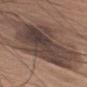biopsy status — no biopsy performed (imaged during a skin exam) | acquisition — 15 mm crop, total-body photography | body site — the right upper arm | subject — male, aged approximately 75.